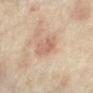– notes — no biopsy performed (imaged during a skin exam)
– TBP lesion metrics — a lesion color around L≈59 a*≈18 b*≈28 in CIELAB, roughly 8 lightness units darker than nearby skin, and a normalized lesion–skin contrast near 5.5; a border-irregularity index near 2.5/10, internal color variation of about 3 on a 0–10 scale, and peripheral color asymmetry of about 1
– patient — female, aged approximately 35
– body site — the arm
– diameter — about 3.5 mm
– illumination — cross-polarized illumination
– image source — ~15 mm crop, total-body skin-cancer survey